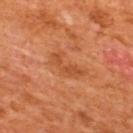  biopsy_status: not biopsied; imaged during a skin examination
  patient:
    sex: male
    age_approx: 65
  automated_metrics:
    area_mm2_approx: 6.5
    eccentricity: 0.9
    shape_asymmetry: 0.55
    border_irregularity_0_10: 6.5
    lesion_detection_confidence_0_100: 100
  site: upper back
  image:
    source: total-body photography crop
    field_of_view_mm: 15
  lighting: cross-polarized
  lesion_size:
    long_diameter_mm_approx: 4.5